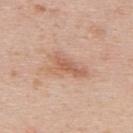No biopsy was performed on this lesion — it was imaged during a full skin examination and was not determined to be concerning. The lesion is located on the upper back. A male patient approximately 40 years of age. This image is a 15 mm lesion crop taken from a total-body photograph. Measured at roughly 5 mm in maximum diameter. This is a white-light tile.